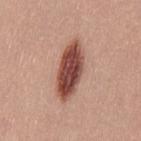Clinical impression: The lesion was tiled from a total-body skin photograph and was not biopsied. Background: Approximately 7 mm at its widest. The total-body-photography lesion software estimated a footprint of about 15 mm², an eccentricity of roughly 0.9, and two-axis asymmetry of about 0.1. And it measured an automated nevus-likeness rating near 100 out of 100 and lesion-presence confidence of about 100/100. A female subject, roughly 25 years of age. The tile uses white-light illumination. On the left thigh. Cropped from a whole-body photographic skin survey; the tile spans about 15 mm.